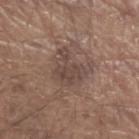* anatomic site · the left forearm
* acquisition · ~15 mm crop, total-body skin-cancer survey
* illumination · white-light
* patient · male, aged around 80
* lesion diameter · ≈4 mm